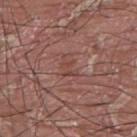workup: imaged on a skin check; not biopsied | TBP lesion metrics: a border-irregularity rating of about 5.5/10 and peripheral color asymmetry of about 0; a nevus-likeness score of about 0/100 | tile lighting: white-light | image: total-body-photography crop, ~15 mm field of view | patient: male, aged approximately 40 | location: the upper back.